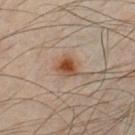illumination=cross-polarized | site=the chest | automated lesion analysis=an area of roughly 5 mm²; a lesion–skin lightness drop of about 12; a border-irregularity rating of about 2.5/10, internal color variation of about 4.5 on a 0–10 scale, and peripheral color asymmetry of about 1; a nevus-likeness score of about 100/100 | patient=male, in their mid- to late 30s | size=≈2.5 mm | imaging modality=total-body-photography crop, ~15 mm field of view.